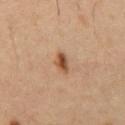Q: Was this lesion biopsied?
A: imaged on a skin check; not biopsied
Q: Where on the body is the lesion?
A: the chest
Q: How was this image acquired?
A: ~15 mm crop, total-body skin-cancer survey
Q: Patient demographics?
A: male, in their mid- to late 50s